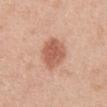Assessment: Imaged during a routine full-body skin examination; the lesion was not biopsied and no histopathology is available. Context: Cropped from a total-body skin-imaging series; the visible field is about 15 mm. A female subject aged approximately 40. The lesion is on the abdomen. This is a white-light tile. Approximately 4.5 mm at its widest.